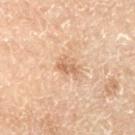Q: Where on the body is the lesion?
A: the right lower leg
Q: Patient demographics?
A: male, aged approximately 70
Q: How was this image acquired?
A: ~15 mm tile from a whole-body skin photo